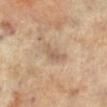Captured during whole-body skin photography for melanoma surveillance; the lesion was not biopsied.
The lesion is located on the right leg.
The subject is a female about 65 years old.
The total-body-photography lesion software estimated a footprint of about 5 mm², a shape eccentricity near 0.8, and two-axis asymmetry of about 0.35. It also reported border irregularity of about 3.5 on a 0–10 scale, a color-variation rating of about 1.5/10, and radial color variation of about 0.5.
The lesion's longest dimension is about 3 mm.
This image is a 15 mm lesion crop taken from a total-body photograph.